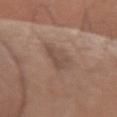This lesion was catalogued during total-body skin photography and was not selected for biopsy. A lesion tile, about 15 mm wide, cut from a 3D total-body photograph. Imaged with white-light lighting. From the left forearm. A male subject, roughly 50 years of age. About 4.5 mm across. An algorithmic analysis of the crop reported a footprint of about 9 mm² and a shape eccentricity near 0.85. The software also gave a lesion-detection confidence of about 100/100.